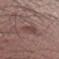The lesion was tiled from a total-body skin photograph and was not biopsied.
Cropped from a whole-body photographic skin survey; the tile spans about 15 mm.
Automated tile analysis of the lesion measured a footprint of about 7.5 mm², a shape eccentricity near 0.95, and a symmetry-axis asymmetry near 0.2. The software also gave a nevus-likeness score of about 0/100 and lesion-presence confidence of about 100/100.
Measured at roughly 5.5 mm in maximum diameter.
Located on the arm.
A male patient, aged approximately 35.